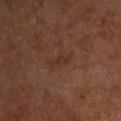notes = no biopsy performed (imaged during a skin exam) | TBP lesion metrics = a footprint of about 2.5 mm² and a shape eccentricity near 0.95; a border-irregularity index near 4/10, a within-lesion color-variation index near 0/10, and peripheral color asymmetry of about 0; a nevus-likeness score of about 0/100 and lesion-presence confidence of about 100/100 | site = the chest | subject = male, aged 58 to 62 | image source = 15 mm crop, total-body photography | illumination = cross-polarized illumination.